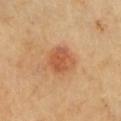| feature | finding |
|---|---|
| biopsy status | no biopsy performed (imaged during a skin exam) |
| patient | female, approximately 70 years of age |
| image source | 15 mm crop, total-body photography |
| body site | the chest |
| diameter | about 3.5 mm |